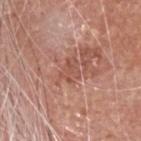– notes — total-body-photography surveillance lesion; no biopsy
– diameter — ≈3 mm
– image — ~15 mm tile from a whole-body skin photo
– automated metrics — a lesion area of about 5 mm² and two-axis asymmetry of about 0.35; a classifier nevus-likeness of about 0/100 and lesion-presence confidence of about 100/100
– illumination — white-light illumination
– patient — male, aged 78–82
– body site — the head or neck